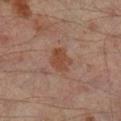No biopsy was performed on this lesion — it was imaged during a full skin examination and was not determined to be concerning. A male patient, in their mid-60s. Measured at roughly 3.5 mm in maximum diameter. A roughly 15 mm field-of-view crop from a total-body skin photograph. Captured under cross-polarized illumination. Located on the right lower leg. The total-body-photography lesion software estimated a footprint of about 7 mm², an outline eccentricity of about 0.65 (0 = round, 1 = elongated), and two-axis asymmetry of about 0.25. The analysis additionally found a border-irregularity index near 3/10 and internal color variation of about 2 on a 0–10 scale.